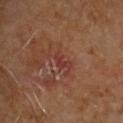  biopsy_status: not biopsied; imaged during a skin examination
  image:
    source: total-body photography crop
    field_of_view_mm: 15
  automated_metrics:
    shape_asymmetry: 0.4
    peripheral_color_asymmetry: 0.0
  patient:
    sex: male
    age_approx: 60
  lighting: cross-polarized
  site: upper back